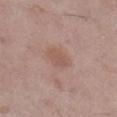Q: Is there a histopathology result?
A: no biopsy performed (imaged during a skin exam)
Q: Who is the patient?
A: female, aged around 60
Q: What is the imaging modality?
A: ~15 mm tile from a whole-body skin photo
Q: Lesion location?
A: the leg
Q: How large is the lesion?
A: ~3 mm (longest diameter)
Q: What lighting was used for the tile?
A: white-light
Q: Automated lesion metrics?
A: a lesion–skin lightness drop of about 7 and a normalized border contrast of about 5.5; an automated nevus-likeness rating near 20 out of 100 and lesion-presence confidence of about 100/100